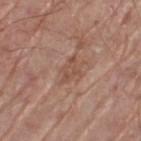Case summary:
– biopsy status — total-body-photography surveillance lesion; no biopsy
– lighting — white-light illumination
– site — the left thigh
– image — total-body-photography crop, ~15 mm field of view
– lesion size — ~3.5 mm (longest diameter)
– patient — male, in their 70s
– image-analysis metrics — an area of roughly 4.5 mm² and an eccentricity of roughly 0.8; a border-irregularity index near 5.5/10, internal color variation of about 1.5 on a 0–10 scale, and radial color variation of about 0.5